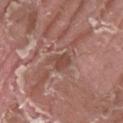Notes:
– follow-up: imaged on a skin check; not biopsied
– lesion size: ~2.5 mm (longest diameter)
– anatomic site: the leg
– patient: male, roughly 40 years of age
– tile lighting: white-light
– image source: 15 mm crop, total-body photography